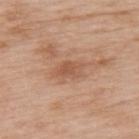{
  "biopsy_status": "not biopsied; imaged during a skin examination",
  "lesion_size": {
    "long_diameter_mm_approx": 3.0
  },
  "site": "upper back",
  "patient": {
    "sex": "female",
    "age_approx": 50
  },
  "automated_metrics": {
    "cielab_L": 54,
    "cielab_a": 23,
    "cielab_b": 32,
    "vs_skin_darker_L": 9.0
  },
  "image": {
    "source": "total-body photography crop",
    "field_of_view_mm": 15
  },
  "lighting": "white-light"
}